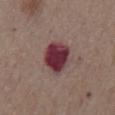notes = catalogued during a skin exam; not biopsied
image = ~15 mm tile from a whole-body skin photo
lesion size = ≈5 mm
location = the chest
image-analysis metrics = a footprint of about 12 mm² and a shape eccentricity near 0.7
patient = male, in their mid-70s